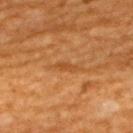Part of a total-body skin-imaging series; this lesion was reviewed on a skin check and was not flagged for biopsy.
Approximately 3 mm at its widest.
A male subject, about 60 years old.
The lesion is located on the back.
The tile uses cross-polarized illumination.
The total-body-photography lesion software estimated an area of roughly 2.5 mm² and a shape eccentricity near 0.95. The analysis additionally found border irregularity of about 3.5 on a 0–10 scale, a within-lesion color-variation index near 0/10, and a peripheral color-asymmetry measure near 0.
Cropped from a total-body skin-imaging series; the visible field is about 15 mm.